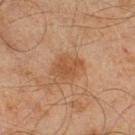Impression: Captured during whole-body skin photography for melanoma surveillance; the lesion was not biopsied. Acquisition and patient details: Imaged with cross-polarized lighting. The subject is a male aged 43–47. The recorded lesion diameter is about 3.5 mm. Located on the right lower leg. A lesion tile, about 15 mm wide, cut from a 3D total-body photograph.